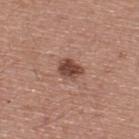Imaged during a routine full-body skin examination; the lesion was not biopsied and no histopathology is available.